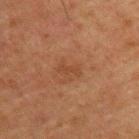Clinical impression: The lesion was photographed on a routine skin check and not biopsied; there is no pathology result. Image and clinical context: The total-body-photography lesion software estimated a shape eccentricity near 0.95 and a symmetry-axis asymmetry near 0.5. It also reported an automated nevus-likeness rating near 0 out of 100 and a lesion-detection confidence of about 100/100. A 15 mm close-up extracted from a 3D total-body photography capture. The lesion is on the upper back. A male subject, aged around 50.